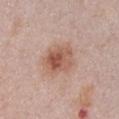biopsy_status: not biopsied; imaged during a skin examination
image:
  source: total-body photography crop
  field_of_view_mm: 15
automated_metrics:
  nevus_likeness_0_100: 95
  lesion_detection_confidence_0_100: 100
lighting: white-light
patient:
  sex: female
  age_approx: 50
lesion_size:
  long_diameter_mm_approx: 4.0
site: front of the torso